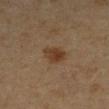The lesion was photographed on a routine skin check and not biopsied; there is no pathology result. Cropped from a whole-body photographic skin survey; the tile spans about 15 mm. A female patient, aged 43–47. Approximately 3 mm at its widest. Automated image analysis of the tile measured about 8 CIELAB-L* units darker than the surrounding skin and a normalized lesion–skin contrast near 8. On the left forearm. Imaged with cross-polarized lighting.